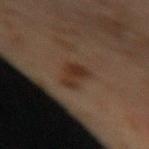Imaged during a routine full-body skin examination; the lesion was not biopsied and no histopathology is available. About 3.5 mm across. The tile uses cross-polarized illumination. A lesion tile, about 15 mm wide, cut from a 3D total-body photograph. The lesion is located on the leg. The patient is a male about 70 years old.